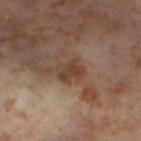Recorded during total-body skin imaging; not selected for excision or biopsy.
Automated image analysis of the tile measured an outline eccentricity of about 0.65 (0 = round, 1 = elongated) and a symmetry-axis asymmetry near 0.2. The software also gave an average lesion color of about L≈42 a*≈18 b*≈28 (CIELAB), roughly 8 lightness units darker than nearby skin, and a normalized lesion–skin contrast near 7.5. It also reported border irregularity of about 2.5 on a 0–10 scale, a within-lesion color-variation index near 3.5/10, and radial color variation of about 1.5. The analysis additionally found a nevus-likeness score of about 0/100 and a lesion-detection confidence of about 100/100.
About 3 mm across.
A close-up tile cropped from a whole-body skin photograph, about 15 mm across.
A female subject roughly 55 years of age.
Located on the left thigh.